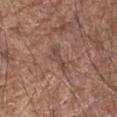Q: Was this lesion biopsied?
A: no biopsy performed (imaged during a skin exam)
Q: Who is the patient?
A: male, aged around 60
Q: How was this image acquired?
A: total-body-photography crop, ~15 mm field of view
Q: Lesion size?
A: about 3.5 mm
Q: What did automated image analysis measure?
A: a footprint of about 4 mm², an eccentricity of roughly 0.9, and a shape-asymmetry score of about 0.45 (0 = symmetric); a lesion color around L≈46 a*≈19 b*≈25 in CIELAB and about 7 CIELAB-L* units darker than the surrounding skin; a border-irregularity index near 5/10 and a within-lesion color-variation index near 0.5/10; lesion-presence confidence of about 85/100
Q: How was the tile lit?
A: white-light illumination
Q: Lesion location?
A: the right forearm